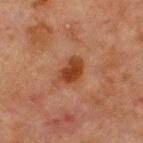Findings:
- site · the front of the torso
- image source · total-body-photography crop, ~15 mm field of view
- subject · male, aged 63–67